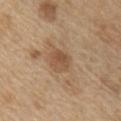imaging modality = 15 mm crop, total-body photography
anatomic site = the right upper arm
tile lighting = white-light illumination
subject = male, about 70 years old